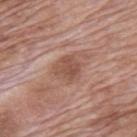Impression: This lesion was catalogued during total-body skin photography and was not selected for biopsy. Acquisition and patient details: On the mid back. About 3 mm across. The tile uses white-light illumination. Cropped from a whole-body photographic skin survey; the tile spans about 15 mm. A male subject approximately 70 years of age.